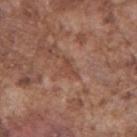The lesion was photographed on a routine skin check and not biopsied; there is no pathology result. About 2.5 mm across. A 15 mm close-up tile from a total-body photography series done for melanoma screening. The lesion is on the right upper arm. Automated tile analysis of the lesion measured an average lesion color of about L≈45 a*≈22 b*≈28 (CIELAB), roughly 7 lightness units darker than nearby skin, and a normalized lesion–skin contrast near 6. It also reported a nevus-likeness score of about 0/100 and a lesion-detection confidence of about 70/100. Captured under white-light illumination. The patient is a male aged approximately 75.